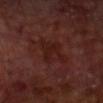Notes:
• biopsy status · no biopsy performed (imaged during a skin exam)
• automated metrics · a lesion area of about 7.5 mm² and a symmetry-axis asymmetry near 0.35; a mean CIELAB color near L≈19 a*≈22 b*≈21, roughly 4 lightness units darker than nearby skin, and a lesion-to-skin contrast of about 6 (normalized; higher = more distinct); a color-variation rating of about 1.5/10 and radial color variation of about 0.5
• subject · male, aged 68–72
• location · the front of the torso
• diameter · ≈3.5 mm
• acquisition · ~15 mm tile from a whole-body skin photo